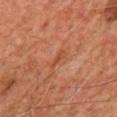Impression: The lesion was photographed on a routine skin check and not biopsied; there is no pathology result. Acquisition and patient details: From the front of the torso. Imaged with cross-polarized lighting. A region of skin cropped from a whole-body photographic capture, roughly 15 mm wide. An algorithmic analysis of the crop reported a border-irregularity rating of about 4.5/10, a color-variation rating of about 0/10, and a peripheral color-asymmetry measure near 0. The analysis additionally found a nevus-likeness score of about 0/100 and a lesion-detection confidence of about 95/100. A male patient, aged approximately 60.